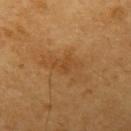<case>
  <biopsy_status>not biopsied; imaged during a skin examination</biopsy_status>
  <automated_metrics>
    <area_mm2_approx>5.0</area_mm2_approx>
    <eccentricity>0.85</eccentricity>
    <shape_asymmetry>0.3</shape_asymmetry>
    <cielab_L>45</cielab_L>
    <cielab_a>21</cielab_a>
    <cielab_b>39</cielab_b>
    <vs_skin_darker_L>6.0</vs_skin_darker_L>
    <vs_skin_contrast_norm>5.0</vs_skin_contrast_norm>
    <color_variation_0_10>2.0</color_variation_0_10>
    <peripheral_color_asymmetry>0.5</peripheral_color_asymmetry>
    <lesion_detection_confidence_0_100>100</lesion_detection_confidence_0_100>
  </automated_metrics>
  <image>
    <source>total-body photography crop</source>
    <field_of_view_mm>15</field_of_view_mm>
  </image>
  <site>left upper arm</site>
  <lesion_size>
    <long_diameter_mm_approx>3.5</long_diameter_mm_approx>
  </lesion_size>
  <lighting>cross-polarized</lighting>
  <patient>
    <sex>male</sex>
    <age_approx>60</age_approx>
  </patient>
</case>